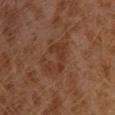| field | value |
|---|---|
| workup | total-body-photography surveillance lesion; no biopsy |
| location | the left leg |
| subject | male, aged 28 to 32 |
| image source | ~15 mm crop, total-body skin-cancer survey |